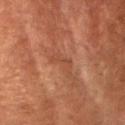Recorded during total-body skin imaging; not selected for excision or biopsy.
The tile uses cross-polarized illumination.
The lesion's longest dimension is about 3 mm.
An algorithmic analysis of the crop reported a shape eccentricity near 0.85 and two-axis asymmetry of about 0.4. It also reported a mean CIELAB color near L≈35 a*≈20 b*≈25, a lesion–skin lightness drop of about 5, and a normalized lesion–skin contrast near 5. The software also gave a border-irregularity rating of about 4.5/10, a within-lesion color-variation index near 1/10, and radial color variation of about 0.
A female subject, aged 78–82.
From the left forearm.
This image is a 15 mm lesion crop taken from a total-body photograph.